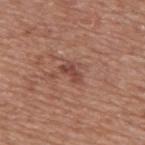Captured during whole-body skin photography for melanoma surveillance; the lesion was not biopsied. The tile uses white-light illumination. A male patient, roughly 75 years of age. Measured at roughly 3 mm in maximum diameter. Automated tile analysis of the lesion measured a lesion color around L≈45 a*≈24 b*≈26 in CIELAB, a lesion–skin lightness drop of about 9, and a lesion-to-skin contrast of about 7 (normalized; higher = more distinct). And it measured a nevus-likeness score of about 0/100. The lesion is located on the upper back. A 15 mm close-up tile from a total-body photography series done for melanoma screening.